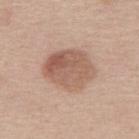No biopsy was performed on this lesion — it was imaged during a full skin examination and was not determined to be concerning. The tile uses white-light illumination. Located on the upper back. A 15 mm close-up tile from a total-body photography series done for melanoma screening. The patient is a male in their 60s. Measured at roughly 5.5 mm in maximum diameter.